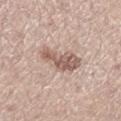Assessment: Part of a total-body skin-imaging series; this lesion was reviewed on a skin check and was not flagged for biopsy. Acquisition and patient details: The lesion is located on the leg. Longest diameter approximately 5 mm. Automated tile analysis of the lesion measured a border-irregularity index near 4.5/10, a color-variation rating of about 4/10, and peripheral color asymmetry of about 1.5. A 15 mm close-up extracted from a 3D total-body photography capture. The subject is a female about 45 years old.